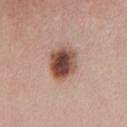Captured during whole-body skin photography for melanoma surveillance; the lesion was not biopsied. About 4 mm across. A 15 mm close-up extracted from a 3D total-body photography capture. The lesion is located on the chest. A female patient, approximately 45 years of age. An algorithmic analysis of the crop reported a mean CIELAB color near L≈48 a*≈21 b*≈27 and a normalized border contrast of about 12.5. And it measured a nevus-likeness score of about 90/100 and a lesion-detection confidence of about 100/100.